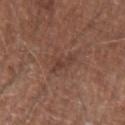Clinical impression: No biopsy was performed on this lesion — it was imaged during a full skin examination and was not determined to be concerning. Background: This is a white-light tile. A 15 mm close-up tile from a total-body photography series done for melanoma screening. The lesion is on the right upper arm. A male patient aged 68 to 72. The total-body-photography lesion software estimated a lesion area of about 4.5 mm², a shape eccentricity near 0.95, and two-axis asymmetry of about 0.4. It also reported a lesion color around L≈39 a*≈20 b*≈24 in CIELAB and a normalized border contrast of about 5.5. It also reported a border-irregularity rating of about 5/10 and a color-variation rating of about 1/10.